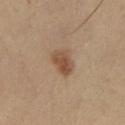Impression:
The lesion was tiled from a total-body skin photograph and was not biopsied.
Background:
A roughly 15 mm field-of-view crop from a total-body skin photograph. A female patient, aged approximately 50. The lesion's longest dimension is about 3 mm. The total-body-photography lesion software estimated a lesion area of about 6 mm² and an outline eccentricity of about 0.55 (0 = round, 1 = elongated). The software also gave a mean CIELAB color near L≈41 a*≈15 b*≈27, roughly 9 lightness units darker than nearby skin, and a normalized border contrast of about 7.5. The analysis additionally found a lesion-detection confidence of about 100/100. The tile uses cross-polarized illumination. The lesion is on the left lower leg.